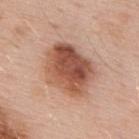Part of a total-body skin-imaging series; this lesion was reviewed on a skin check and was not flagged for biopsy. A male subject, about 55 years old. The tile uses white-light illumination. The lesion is located on the upper back. An algorithmic analysis of the crop reported an eccentricity of roughly 0.65 and two-axis asymmetry of about 0.2. And it measured an average lesion color of about L≈53 a*≈24 b*≈31 (CIELAB) and roughly 15 lightness units darker than nearby skin. The analysis additionally found internal color variation of about 9 on a 0–10 scale. It also reported an automated nevus-likeness rating near 95 out of 100 and a lesion-detection confidence of about 100/100. A 15 mm crop from a total-body photograph taken for skin-cancer surveillance. The lesion's longest dimension is about 6.5 mm.